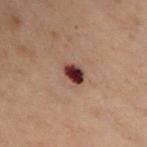workup = imaged on a skin check; not biopsied | image source = ~15 mm tile from a whole-body skin photo | subject = male, aged 48–52 | body site = the chest.